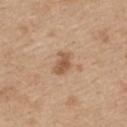{"biopsy_status": "not biopsied; imaged during a skin examination", "lesion_size": {"long_diameter_mm_approx": 3.0}, "image": {"source": "total-body photography crop", "field_of_view_mm": 15}, "lighting": "white-light", "site": "upper back", "patient": {"sex": "female", "age_approx": 45}}